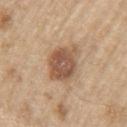Impression: Part of a total-body skin-imaging series; this lesion was reviewed on a skin check and was not flagged for biopsy. Acquisition and patient details: On the left upper arm. A 15 mm close-up extracted from a 3D total-body photography capture. A male subject roughly 70 years of age.